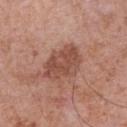Imaged during a routine full-body skin examination; the lesion was not biopsied and no histopathology is available. This image is a 15 mm lesion crop taken from a total-body photograph. An algorithmic analysis of the crop reported an area of roughly 13 mm², a shape eccentricity near 0.7, and a symmetry-axis asymmetry near 0.2. It also reported a lesion–skin lightness drop of about 10 and a normalized lesion–skin contrast near 7.5. The analysis additionally found a nevus-likeness score of about 0/100 and a detector confidence of about 100 out of 100 that the crop contains a lesion. The lesion is on the chest. A male subject aged around 70.